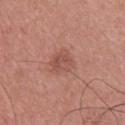{"biopsy_status": "not biopsied; imaged during a skin examination", "site": "upper back", "lesion_size": {"long_diameter_mm_approx": 3.0}, "patient": {"sex": "female", "age_approx": 40}, "lighting": "white-light", "image": {"source": "total-body photography crop", "field_of_view_mm": 15}}